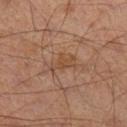A 15 mm crop from a total-body photograph taken for skin-cancer surveillance. The tile uses cross-polarized illumination. From the right lower leg. A male patient approximately 65 years of age. The lesion-visualizer software estimated a within-lesion color-variation index near 0/10 and peripheral color asymmetry of about 0. It also reported a nevus-likeness score of about 5/100 and lesion-presence confidence of about 100/100.